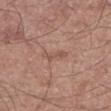Assessment: Part of a total-body skin-imaging series; this lesion was reviewed on a skin check and was not flagged for biopsy. Context: The lesion is located on the left thigh. This is a white-light tile. A male patient about 55 years old. A region of skin cropped from a whole-body photographic capture, roughly 15 mm wide. The lesion's longest dimension is about 3 mm.